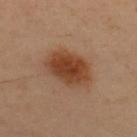follow-up: total-body-photography surveillance lesion; no biopsy | body site: the back | patient: male, aged 43–47 | TBP lesion metrics: an average lesion color of about L≈41 a*≈22 b*≈32 (CIELAB), about 12 CIELAB-L* units darker than the surrounding skin, and a lesion-to-skin contrast of about 10 (normalized; higher = more distinct); a classifier nevus-likeness of about 100/100 and a lesion-detection confidence of about 100/100 | illumination: cross-polarized | lesion size: ≈5.5 mm | imaging modality: 15 mm crop, total-body photography.